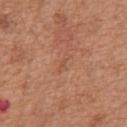This lesion was catalogued during total-body skin photography and was not selected for biopsy. Cropped from a whole-body photographic skin survey; the tile spans about 15 mm. Captured under white-light illumination. The subject is a male aged approximately 65. About 1 mm across. From the abdomen.